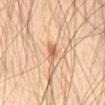Part of a total-body skin-imaging series; this lesion was reviewed on a skin check and was not flagged for biopsy.
Captured under cross-polarized illumination.
A male subject aged 58–62.
Located on the abdomen.
A 15 mm crop from a total-body photograph taken for skin-cancer surveillance.
The lesion-visualizer software estimated a border-irregularity index near 3.5/10, a within-lesion color-variation index near 3.5/10, and radial color variation of about 1.